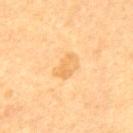Notes:
– notes — no biopsy performed (imaged during a skin exam)
– diameter — ≈3.5 mm
– automated metrics — a mean CIELAB color near L≈72 a*≈20 b*≈46, about 7 CIELAB-L* units darker than the surrounding skin, and a normalized border contrast of about 5; a peripheral color-asymmetry measure near 1
– subject — male, roughly 60 years of age
– image source — ~15 mm tile from a whole-body skin photo
– location — the mid back
– illumination — cross-polarized